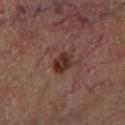Part of a total-body skin-imaging series; this lesion was reviewed on a skin check and was not flagged for biopsy. A region of skin cropped from a whole-body photographic capture, roughly 15 mm wide. The lesion's longest dimension is about 2.5 mm. Imaged with cross-polarized lighting. From the leg. A male patient in their mid-60s. Automated tile analysis of the lesion measured a lesion area of about 5 mm², an eccentricity of roughly 0.6, and two-axis asymmetry of about 0.2. And it measured internal color variation of about 5 on a 0–10 scale and a peripheral color-asymmetry measure near 2.